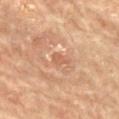Impression: Captured during whole-body skin photography for melanoma surveillance; the lesion was not biopsied. Background: Measured at roughly 2.5 mm in maximum diameter. The patient is a female aged approximately 80. From the back. Cropped from a whole-body photographic skin survey; the tile spans about 15 mm.